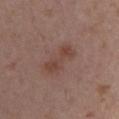Impression:
Part of a total-body skin-imaging series; this lesion was reviewed on a skin check and was not flagged for biopsy.
Image and clinical context:
Cropped from a total-body skin-imaging series; the visible field is about 15 mm. The lesion is on the back. A female patient, about 30 years old.